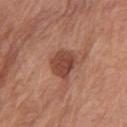Impression: The lesion was photographed on a routine skin check and not biopsied; there is no pathology result. Background: The lesion is on the arm. The total-body-photography lesion software estimated an area of roughly 8.5 mm² and a symmetry-axis asymmetry near 0.25. It also reported a lesion color around L≈45 a*≈24 b*≈28 in CIELAB, about 12 CIELAB-L* units darker than the surrounding skin, and a lesion-to-skin contrast of about 8.5 (normalized; higher = more distinct). It also reported a border-irregularity index near 2.5/10 and a within-lesion color-variation index near 3/10. A female patient in their mid-60s. A region of skin cropped from a whole-body photographic capture, roughly 15 mm wide.